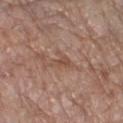Recorded during total-body skin imaging; not selected for excision or biopsy.
Captured under white-light illumination.
On the left lower leg.
The subject is a female approximately 85 years of age or older.
A 15 mm crop from a total-body photograph taken for skin-cancer surveillance.
Longest diameter approximately 3.5 mm.